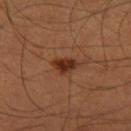biopsy status — imaged on a skin check; not biopsied
automated metrics — a shape eccentricity near 0.8 and a shape-asymmetry score of about 0.35 (0 = symmetric); a lesion–skin lightness drop of about 11 and a normalized border contrast of about 10; a border-irregularity rating of about 3/10, a color-variation rating of about 3/10, and a peripheral color-asymmetry measure near 1
patient — male, in their 50s
illumination — cross-polarized
lesion size — ~3.5 mm (longest diameter)
body site — the right thigh
image — ~15 mm tile from a whole-body skin photo